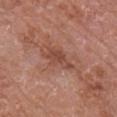{"biopsy_status": "not biopsied; imaged during a skin examination", "lesion_size": {"long_diameter_mm_approx": 4.0}, "lighting": "white-light", "site": "right upper arm", "image": {"source": "total-body photography crop", "field_of_view_mm": 15}, "patient": {"sex": "male", "age_approx": 65}}